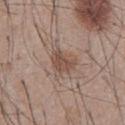- size: about 3 mm
- illumination: white-light illumination
- subject: male, aged 53 to 57
- image source: ~15 mm crop, total-body skin-cancer survey
- automated lesion analysis: an eccentricity of roughly 0.6 and two-axis asymmetry of about 0.35
- site: the chest